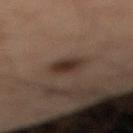The lesion was tiled from a total-body skin photograph and was not biopsied.
Imaged with cross-polarized lighting.
The patient is a male aged approximately 50.
Automated image analysis of the tile measured a mean CIELAB color near L≈30 a*≈15 b*≈21. The analysis additionally found a border-irregularity index near 1.5/10 and internal color variation of about 2.5 on a 0–10 scale. The analysis additionally found a nevus-likeness score of about 95/100 and lesion-presence confidence of about 100/100.
A 15 mm close-up extracted from a 3D total-body photography capture.
Located on the left leg.
Longest diameter approximately 3 mm.